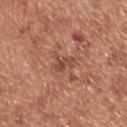Findings:
* notes — total-body-photography surveillance lesion; no biopsy
* acquisition — 15 mm crop, total-body photography
* lesion diameter — ≈2.5 mm
* site — the upper back
* illumination — white-light
* subject — male, aged 63–67